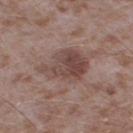| feature | finding |
|---|---|
| biopsy status | no biopsy performed (imaged during a skin exam) |
| site | the right thigh |
| lesion size | ≈6.5 mm |
| subject | male, in their mid- to late 40s |
| imaging modality | 15 mm crop, total-body photography |
| automated lesion analysis | an area of roughly 16 mm², a shape eccentricity near 0.8, and two-axis asymmetry of about 0.3; a lesion color around L≈45 a*≈18 b*≈21 in CIELAB, a lesion–skin lightness drop of about 10, and a lesion-to-skin contrast of about 7.5 (normalized; higher = more distinct); a classifier nevus-likeness of about 70/100 and a lesion-detection confidence of about 100/100 |